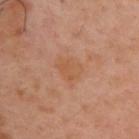Captured during whole-body skin photography for melanoma surveillance; the lesion was not biopsied. Approximately 3.5 mm at its widest. A 15 mm close-up tile from a total-body photography series done for melanoma screening. The lesion-visualizer software estimated a border-irregularity index near 3/10, a within-lesion color-variation index near 2/10, and peripheral color asymmetry of about 1. It also reported a lesion-detection confidence of about 100/100. A male patient aged around 40. The lesion is on the back. Imaged with cross-polarized lighting.